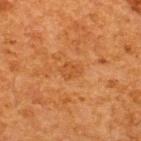<tbp_lesion>
<biopsy_status>not biopsied; imaged during a skin examination</biopsy_status>
<site>back</site>
<image>
  <source>total-body photography crop</source>
  <field_of_view_mm>15</field_of_view_mm>
</image>
<lighting>cross-polarized</lighting>
<lesion_size>
  <long_diameter_mm_approx>2.5</long_diameter_mm_approx>
</lesion_size>
<automated_metrics>
  <cielab_L>42</cielab_L>
  <cielab_a>24</cielab_a>
  <cielab_b>38</cielab_b>
  <vs_skin_darker_L>5.0</vs_skin_darker_L>
  <border_irregularity_0_10>2.5</border_irregularity_0_10>
  <color_variation_0_10>1.0</color_variation_0_10>
  <peripheral_color_asymmetry>0.5</peripheral_color_asymmetry>
  <nevus_likeness_0_100>0</nevus_likeness_0_100>
</automated_metrics>
<patient>
  <sex>male</sex>
  <age_approx>65</age_approx>
</patient>
</tbp_lesion>